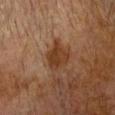Assessment:
Imaged during a routine full-body skin examination; the lesion was not biopsied and no histopathology is available.
Acquisition and patient details:
Cropped from a total-body skin-imaging series; the visible field is about 15 mm. About 4 mm across. A male patient, aged approximately 75. The lesion is on the head or neck.